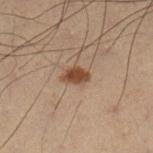| key | value |
|---|---|
| follow-up | total-body-photography surveillance lesion; no biopsy |
| image source | ~15 mm crop, total-body skin-cancer survey |
| subject | male, aged 53 to 57 |
| lighting | cross-polarized |
| site | the left lower leg |